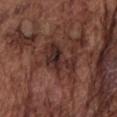Recorded during total-body skin imaging; not selected for excision or biopsy.
Cropped from a whole-body photographic skin survey; the tile spans about 15 mm.
The total-body-photography lesion software estimated a shape-asymmetry score of about 0.55 (0 = symmetric).
The lesion's longest dimension is about 5 mm.
This is a white-light tile.
A male patient roughly 75 years of age.
The lesion is located on the chest.